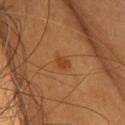biopsy_status: not biopsied; imaged during a skin examination
site: head or neck
patient:
  sex: female
  age_approx: 55
automated_metrics:
  area_mm2_approx: 3.0
  shape_asymmetry: 0.35
  border_irregularity_0_10: 3.0
  color_variation_0_10: 1.0
  peripheral_color_asymmetry: 0.5
  nevus_likeness_0_100: 85
  lesion_detection_confidence_0_100: 100
lesion_size:
  long_diameter_mm_approx: 2.0
image:
  source: total-body photography crop
  field_of_view_mm: 15